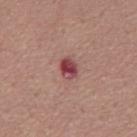  biopsy_status: not biopsied; imaged during a skin examination
  patient:
    sex: male
    age_approx: 45
  automated_metrics:
    area_mm2_approx: 4.5
    eccentricity: 0.65
    shape_asymmetry: 0.2
    cielab_L: 45
    cielab_a: 29
    cielab_b: 21
    vs_skin_darker_L: 14.0
    vs_skin_contrast_norm: 10.5
    border_irregularity_0_10: 2.0
    color_variation_0_10: 6.0
    peripheral_color_asymmetry: 2.0
  site: mid back
  image:
    source: total-body photography crop
    field_of_view_mm: 15
  lighting: white-light
  lesion_size:
    long_diameter_mm_approx: 2.5